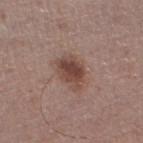image — ~15 mm crop, total-body skin-cancer survey
patient — male, aged approximately 65
location — the left lower leg